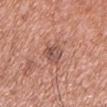Recorded during total-body skin imaging; not selected for excision or biopsy.
About 4 mm across.
Located on the left upper arm.
A 15 mm crop from a total-body photograph taken for skin-cancer surveillance.
Imaged with white-light lighting.
A male patient, aged 63 to 67.